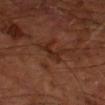Image and clinical context:
Cropped from a whole-body photographic skin survey; the tile spans about 15 mm. The recorded lesion diameter is about 3 mm. This is a cross-polarized tile. From the left forearm. A male subject, in their mid- to late 60s.